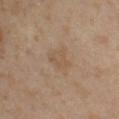Assessment: The lesion was tiled from a total-body skin photograph and was not biopsied. Context: This is a cross-polarized tile. A roughly 15 mm field-of-view crop from a total-body skin photograph. Located on the left upper arm. A female patient aged 48–52. The lesion's longest dimension is about 3.5 mm.